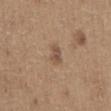biopsy status: imaged on a skin check; not biopsied | patient: male, approximately 65 years of age | lesion diameter: ≈2.5 mm | image: ~15 mm crop, total-body skin-cancer survey | TBP lesion metrics: an area of roughly 3.5 mm², an outline eccentricity of about 0.8 (0 = round, 1 = elongated), and two-axis asymmetry of about 0.2; an average lesion color of about L≈51 a*≈17 b*≈28 (CIELAB) and a normalized lesion–skin contrast near 6.5; a border-irregularity rating of about 2/10 and a within-lesion color-variation index near 2/10; a lesion-detection confidence of about 100/100 | anatomic site: the abdomen.